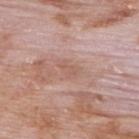| field | value |
|---|---|
| diameter | about 2.5 mm |
| imaging modality | ~15 mm tile from a whole-body skin photo |
| anatomic site | the back |
| patient | male, in their 60s |
| illumination | white-light illumination |
| automated metrics | a footprint of about 3.5 mm² and a shape eccentricity near 0.8; a color-variation rating of about 1.5/10; a nevus-likeness score of about 0/100 and a lesion-detection confidence of about 100/100 |